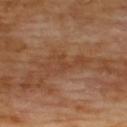{
  "biopsy_status": "not biopsied; imaged during a skin examination",
  "patient": {
    "sex": "male",
    "age_approx": 70
  },
  "lighting": "cross-polarized",
  "lesion_size": {
    "long_diameter_mm_approx": 5.5
  },
  "site": "upper back",
  "image": {
    "source": "total-body photography crop",
    "field_of_view_mm": 15
  }
}